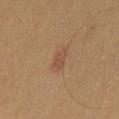Clinical impression: This lesion was catalogued during total-body skin photography and was not selected for biopsy. Acquisition and patient details: The lesion is on the left thigh. A lesion tile, about 15 mm wide, cut from a 3D total-body photograph. A female patient aged approximately 50. This is a cross-polarized tile. Measured at roughly 3 mm in maximum diameter.